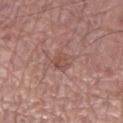– workup · no biopsy performed (imaged during a skin exam)
– size · about 3 mm
– patient · male, aged around 70
– acquisition · ~15 mm crop, total-body skin-cancer survey
– illumination · white-light
– location · the left lower leg
– TBP lesion metrics · a footprint of about 6 mm² and an eccentricity of roughly 0.65; a within-lesion color-variation index near 3.5/10 and peripheral color asymmetry of about 1